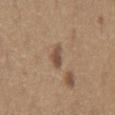<lesion>
  <biopsy_status>not biopsied; imaged during a skin examination</biopsy_status>
  <lighting>white-light</lighting>
  <image>
    <source>total-body photography crop</source>
    <field_of_view_mm>15</field_of_view_mm>
  </image>
  <site>chest</site>
  <patient>
    <sex>male</sex>
    <age_approx>65</age_approx>
  </patient>
  <lesion_size>
    <long_diameter_mm_approx>3.0</long_diameter_mm_approx>
  </lesion_size>
  <automated_metrics>
    <cielab_L>49</cielab_L>
    <cielab_a>16</cielab_a>
    <cielab_b>28</cielab_b>
    <border_irregularity_0_10>4.0</border_irregularity_0_10>
    <color_variation_0_10>1.5</color_variation_0_10>
    <peripheral_color_asymmetry>0.5</peripheral_color_asymmetry>
    <lesion_detection_confidence_0_100>100</lesion_detection_confidence_0_100>
  </automated_metrics>
</lesion>